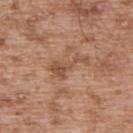Findings:
• follow-up · catalogued during a skin exam; not biopsied
• lighting · white-light illumination
• size · about 5 mm
• subject · male, aged 53 to 57
• image source · ~15 mm crop, total-body skin-cancer survey
• location · the upper back